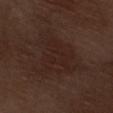biopsy_status: not biopsied; imaged during a skin examination
patient:
  sex: male
  age_approx: 70
image:
  source: total-body photography crop
  field_of_view_mm: 15
site: leg